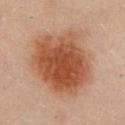{
  "site": "chest",
  "patient": {
    "sex": "female",
    "age_approx": 35
  },
  "lighting": "cross-polarized",
  "lesion_size": {
    "long_diameter_mm_approx": 8.0
  },
  "image": {
    "source": "total-body photography crop",
    "field_of_view_mm": 15
  }
}